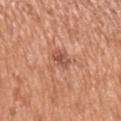The lesion was photographed on a routine skin check and not biopsied; there is no pathology result. A roughly 15 mm field-of-view crop from a total-body skin photograph. Located on the left upper arm. A female patient aged 73–77.